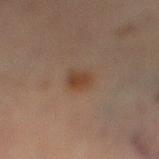biopsy status: catalogued during a skin exam; not biopsied
acquisition: 15 mm crop, total-body photography
automated lesion analysis: an area of roughly 5 mm² and a shape-asymmetry score of about 0.2 (0 = symmetric); a nevus-likeness score of about 90/100
diameter: about 3 mm
tile lighting: cross-polarized
location: the right lower leg
patient: female, about 70 years old